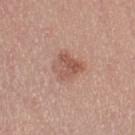Imaged during a routine full-body skin examination; the lesion was not biopsied and no histopathology is available.
A region of skin cropped from a whole-body photographic capture, roughly 15 mm wide.
Longest diameter approximately 3.5 mm.
The subject is a female aged around 35.
Located on the left thigh.